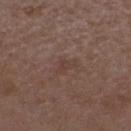<tbp_lesion>
<biopsy_status>not biopsied; imaged during a skin examination</biopsy_status>
<image>
  <source>total-body photography crop</source>
  <field_of_view_mm>15</field_of_view_mm>
</image>
<patient>
  <sex>female</sex>
  <age_approx>35</age_approx>
</patient>
<lighting>white-light</lighting>
<lesion_size>
  <long_diameter_mm_approx>3.0</long_diameter_mm_approx>
</lesion_size>
<automated_metrics>
  <area_mm2_approx>2.5</area_mm2_approx>
  <eccentricity>0.85</eccentricity>
  <shape_asymmetry>0.5</shape_asymmetry>
</automated_metrics>
<site>head or neck</site>
</tbp_lesion>